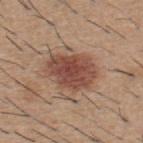workup = total-body-photography surveillance lesion; no biopsy | site = the upper back | size = ~6 mm (longest diameter) | automated metrics = a nevus-likeness score of about 100/100 and lesion-presence confidence of about 100/100 | acquisition = ~15 mm tile from a whole-body skin photo | patient = male, about 60 years old.